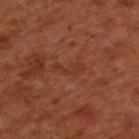No biopsy was performed on this lesion — it was imaged during a full skin examination and was not determined to be concerning. A lesion tile, about 15 mm wide, cut from a 3D total-body photograph. Measured at roughly 3.5 mm in maximum diameter. The patient is a male aged 48–52. This is a cross-polarized tile. From the upper back.